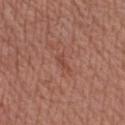Clinical impression: Part of a total-body skin-imaging series; this lesion was reviewed on a skin check and was not flagged for biopsy. Clinical summary: Located on the left forearm. The subject is a male approximately 55 years of age. A region of skin cropped from a whole-body photographic capture, roughly 15 mm wide.